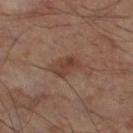Clinical impression:
Captured during whole-body skin photography for melanoma surveillance; the lesion was not biopsied.
Background:
This image is a 15 mm lesion crop taken from a total-body photograph. This is a cross-polarized tile. The lesion's longest dimension is about 4 mm. The lesion-visualizer software estimated a lesion area of about 8 mm², a shape eccentricity near 0.8, and two-axis asymmetry of about 0.25. And it measured border irregularity of about 2.5 on a 0–10 scale and a color-variation rating of about 3/10. The software also gave a detector confidence of about 100 out of 100 that the crop contains a lesion. A male subject, roughly 55 years of age. The lesion is located on the left lower leg.